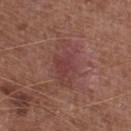A male patient, in their mid- to late 70s.
A close-up tile cropped from a whole-body skin photograph, about 15 mm across.
On the leg.